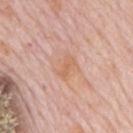workup = total-body-photography surveillance lesion; no biopsy
lesion size = ≈2.5 mm
patient = male, roughly 80 years of age
tile lighting = white-light illumination
body site = the upper back
acquisition = 15 mm crop, total-body photography